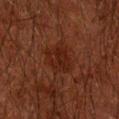A male subject, aged 58 to 62. About 3.5 mm across. From the left forearm. Automated tile analysis of the lesion measured peripheral color asymmetry of about 1. A region of skin cropped from a whole-body photographic capture, roughly 15 mm wide.